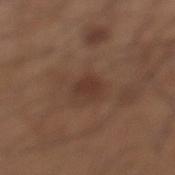notes = no biopsy performed (imaged during a skin exam) | diameter = about 2.5 mm | anatomic site = the left lower leg | image = ~15 mm crop, total-body skin-cancer survey | subject = male, about 60 years old | illumination = white-light.